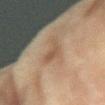Clinical impression:
Imaged during a routine full-body skin examination; the lesion was not biopsied and no histopathology is available.
Context:
A female patient, aged 78 to 82. Cropped from a total-body skin-imaging series; the visible field is about 15 mm. From the arm. This is a cross-polarized tile.